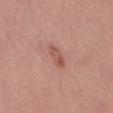Imaged during a routine full-body skin examination; the lesion was not biopsied and no histopathology is available. The lesion is on the leg. The lesion's longest dimension is about 3.5 mm. An algorithmic analysis of the crop reported a footprint of about 4 mm² and a shape eccentricity near 0.9. And it measured a mean CIELAB color near L≈54 a*≈25 b*≈26, roughly 9 lightness units darker than nearby skin, and a lesion-to-skin contrast of about 6 (normalized; higher = more distinct). The analysis additionally found border irregularity of about 2.5 on a 0–10 scale, a within-lesion color-variation index near 4/10, and a peripheral color-asymmetry measure near 1.5. It also reported a nevus-likeness score of about 35/100 and lesion-presence confidence of about 100/100. A close-up tile cropped from a whole-body skin photograph, about 15 mm across. The tile uses white-light illumination. The patient is a female aged 33–37.